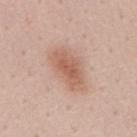<record>
  <site>mid back</site>
  <patient>
    <sex>male</sex>
    <age_approx>55</age_approx>
  </patient>
  <lighting>white-light</lighting>
  <image>
    <source>total-body photography crop</source>
    <field_of_view_mm>15</field_of_view_mm>
  </image>
  <lesion_size>
    <long_diameter_mm_approx>5.0</long_diameter_mm_approx>
  </lesion_size>
</record>